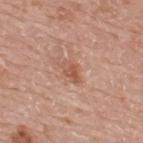workup: imaged on a skin check; not biopsied